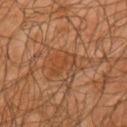Assessment: Imaged during a routine full-body skin examination; the lesion was not biopsied and no histopathology is available. Acquisition and patient details: A roughly 15 mm field-of-view crop from a total-body skin photograph. Imaged with cross-polarized lighting. A male subject aged approximately 65. On the right upper arm. Measured at roughly 3.5 mm in maximum diameter.